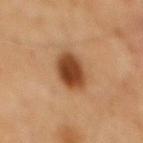notes: catalogued during a skin exam; not biopsied | location: the back | automated metrics: an area of roughly 11 mm², an eccentricity of roughly 0.6, and two-axis asymmetry of about 0.1; a nevus-likeness score of about 100/100 and a lesion-detection confidence of about 100/100 | subject: male, aged approximately 65 | image source: total-body-photography crop, ~15 mm field of view.